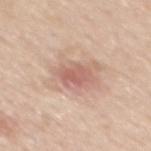No biopsy was performed on this lesion — it was imaged during a full skin examination and was not determined to be concerning.
The lesion-visualizer software estimated a shape eccentricity near 0.7 and a shape-asymmetry score of about 0.25 (0 = symmetric). And it measured lesion-presence confidence of about 100/100.
Imaged with white-light lighting.
Longest diameter approximately 3.5 mm.
A 15 mm crop from a total-body photograph taken for skin-cancer surveillance.
A male subject approximately 50 years of age.
On the mid back.